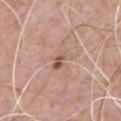{
  "biopsy_status": "not biopsied; imaged during a skin examination",
  "patient": {
    "sex": "male",
    "age_approx": 50
  },
  "image": {
    "source": "total-body photography crop",
    "field_of_view_mm": 15
  },
  "site": "chest"
}